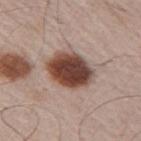biopsy status: no biopsy performed (imaged during a skin exam) | patient: male, aged around 65 | diameter: ≈5.5 mm | acquisition: ~15 mm crop, total-body skin-cancer survey | lighting: white-light | site: the right upper arm.